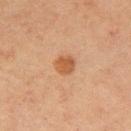The lesion was photographed on a routine skin check and not biopsied; there is no pathology result. This is a cross-polarized tile. A male patient aged 58–62. The lesion's longest dimension is about 2.5 mm. A roughly 15 mm field-of-view crop from a total-body skin photograph. From the right upper arm.